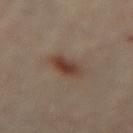Imaged during a routine full-body skin examination; the lesion was not biopsied and no histopathology is available. A 15 mm close-up extracted from a 3D total-body photography capture. A female patient in their 60s. From the back.